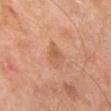Background:
This is a cross-polarized tile. A male subject, about 50 years old. From the left leg. Measured at roughly 3 mm in maximum diameter. A roughly 15 mm field-of-view crop from a total-body skin photograph.